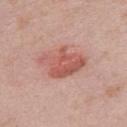{
  "biopsy_status": "not biopsied; imaged during a skin examination",
  "automated_metrics": {
    "vs_skin_darker_L": 11.0,
    "border_irregularity_0_10": 3.5,
    "peripheral_color_asymmetry": 2.0
  },
  "site": "right upper arm",
  "lesion_size": {
    "long_diameter_mm_approx": 5.5
  },
  "image": {
    "source": "total-body photography crop",
    "field_of_view_mm": 15
  },
  "patient": {
    "sex": "male",
    "age_approx": 40
  },
  "lighting": "white-light"
}